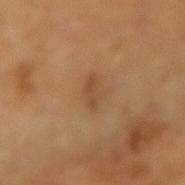Clinical impression:
Captured during whole-body skin photography for melanoma surveillance; the lesion was not biopsied.
Image and clinical context:
This image is a 15 mm lesion crop taken from a total-body photograph. A female subject, in their mid-50s. From the left forearm.